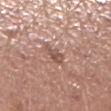{"biopsy_status": "not biopsied; imaged during a skin examination", "image": {"source": "total-body photography crop", "field_of_view_mm": 15}, "automated_metrics": {"eccentricity": 0.85, "shape_asymmetry": 0.3, "cielab_L": 52, "cielab_a": 20, "cielab_b": 25, "vs_skin_contrast_norm": 7.0, "color_variation_0_10": 2.0, "peripheral_color_asymmetry": 0.5, "nevus_likeness_0_100": 0, "lesion_detection_confidence_0_100": 90}, "lesion_size": {"long_diameter_mm_approx": 3.0}, "patient": {"sex": "female", "age_approx": 65}, "site": "leg"}